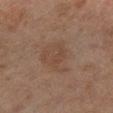biopsy status: catalogued during a skin exam; not biopsied | site: the left lower leg | tile lighting: cross-polarized | lesion diameter: ~5.5 mm (longest diameter) | image: total-body-photography crop, ~15 mm field of view | patient: female, roughly 60 years of age | image-analysis metrics: an average lesion color of about L≈39 a*≈14 b*≈24 (CIELAB), a lesion–skin lightness drop of about 5, and a normalized border contrast of about 4.5; a border-irregularity index near 3.5/10, a color-variation rating of about 2.5/10, and radial color variation of about 0.5; an automated nevus-likeness rating near 5 out of 100 and a detector confidence of about 100 out of 100 that the crop contains a lesion.